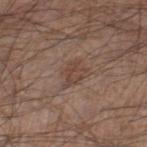Captured during whole-body skin photography for melanoma surveillance; the lesion was not biopsied.